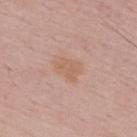follow-up: total-body-photography surveillance lesion; no biopsy
tile lighting: white-light illumination
body site: the upper back
imaging modality: ~15 mm crop, total-body skin-cancer survey
lesion size: ≈3.5 mm
automated metrics: an area of roughly 6 mm², an outline eccentricity of about 0.7 (0 = round, 1 = elongated), and two-axis asymmetry of about 0.25; a mean CIELAB color near L≈61 a*≈19 b*≈30 and about 6 CIELAB-L* units darker than the surrounding skin
subject: male, aged 48–52